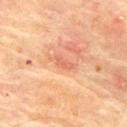Part of a total-body skin-imaging series; this lesion was reviewed on a skin check and was not flagged for biopsy. Automated image analysis of the tile measured a lesion area of about 2.5 mm² and a shape eccentricity near 0.9. It also reported a lesion color around L≈55 a*≈25 b*≈32 in CIELAB, about 7 CIELAB-L* units darker than the surrounding skin, and a normalized border contrast of about 5. It also reported a nevus-likeness score of about 0/100 and lesion-presence confidence of about 100/100. The patient is a male approximately 75 years of age. Cropped from a total-body skin-imaging series; the visible field is about 15 mm. The lesion is on the upper back.